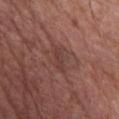Imaged during a routine full-body skin examination; the lesion was not biopsied and no histopathology is available.